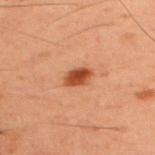This lesion was catalogued during total-body skin photography and was not selected for biopsy. About 3 mm across. The lesion-visualizer software estimated a footprint of about 4.5 mm² and an outline eccentricity of about 0.8 (0 = round, 1 = elongated). It also reported border irregularity of about 1.5 on a 0–10 scale and a within-lesion color-variation index near 3/10. The analysis additionally found a classifier nevus-likeness of about 100/100 and a lesion-detection confidence of about 100/100. Located on the back. This is a cross-polarized tile. A male patient roughly 60 years of age. Cropped from a total-body skin-imaging series; the visible field is about 15 mm.